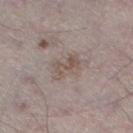Captured during whole-body skin photography for melanoma surveillance; the lesion was not biopsied. An algorithmic analysis of the crop reported a color-variation rating of about 1/10 and radial color variation of about 0. The software also gave a lesion-detection confidence of about 60/100. Cropped from a total-body skin-imaging series; the visible field is about 15 mm. The patient is a male aged 68–72. On the leg. Captured under white-light illumination.